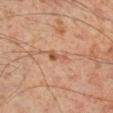{"biopsy_status": "not biopsied; imaged during a skin examination", "site": "right lower leg", "automated_metrics": {"border_irregularity_0_10": 6.0, "color_variation_0_10": 0.0, "peripheral_color_asymmetry": 0.0, "nevus_likeness_0_100": 5, "lesion_detection_confidence_0_100": 100}, "lesion_size": {"long_diameter_mm_approx": 2.5}, "image": {"source": "total-body photography crop", "field_of_view_mm": 15}, "patient": {"sex": "male", "age_approx": 55}}